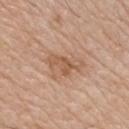No biopsy was performed on this lesion — it was imaged during a full skin examination and was not determined to be concerning.
Imaged with white-light lighting.
The lesion is located on the chest.
A roughly 15 mm field-of-view crop from a total-body skin photograph.
About 4.5 mm across.
A male subject, aged approximately 65.